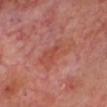Recorded during total-body skin imaging; not selected for excision or biopsy. The lesion is on the head or neck. The lesion's longest dimension is about 4.5 mm. A roughly 15 mm field-of-view crop from a total-body skin photograph. This is a cross-polarized tile. A male patient aged 63–67.